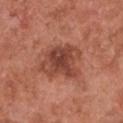  biopsy_status: not biopsied; imaged during a skin examination
  site: chest
  lighting: white-light
  patient:
    sex: female
    age_approx: 50
  automated_metrics:
    area_mm2_approx: 16.0
    eccentricity: 0.6
    shape_asymmetry: 0.3
  lesion_size:
    long_diameter_mm_approx: 5.0
  image:
    source: total-body photography crop
    field_of_view_mm: 15